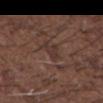Assessment: Imaged during a routine full-body skin examination; the lesion was not biopsied and no histopathology is available. Acquisition and patient details: The total-body-photography lesion software estimated roughly 6 lightness units darker than nearby skin and a normalized border contrast of about 5.5. The recorded lesion diameter is about 2.5 mm. Located on the upper back. The subject is a male aged around 50. A lesion tile, about 15 mm wide, cut from a 3D total-body photograph. This is a white-light tile.